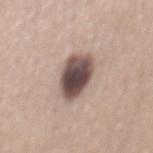biopsy_status: not biopsied; imaged during a skin examination
lighting: white-light
site: mid back
image:
  source: total-body photography crop
  field_of_view_mm: 15
lesion_size:
  long_diameter_mm_approx: 5.0
patient:
  sex: male
  age_approx: 55